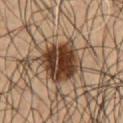Recorded during total-body skin imaging; not selected for excision or biopsy. From the chest. Imaged with cross-polarized lighting. About 5 mm across. A 15 mm close-up tile from a total-body photography series done for melanoma screening. The subject is a male approximately 50 years of age.